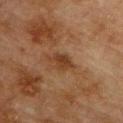Part of a total-body skin-imaging series; this lesion was reviewed on a skin check and was not flagged for biopsy.
A male patient in their mid-70s.
The lesion is located on the chest.
Captured under cross-polarized illumination.
A 15 mm close-up tile from a total-body photography series done for melanoma screening.
About 3.5 mm across.